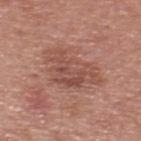Q: Was a biopsy performed?
A: imaged on a skin check; not biopsied
Q: Lesion size?
A: ~7 mm (longest diameter)
Q: What is the imaging modality?
A: ~15 mm tile from a whole-body skin photo
Q: What did automated image analysis measure?
A: a border-irregularity rating of about 6/10, internal color variation of about 4 on a 0–10 scale, and peripheral color asymmetry of about 1.5; a nevus-likeness score of about 0/100 and a detector confidence of about 100 out of 100 that the crop contains a lesion
Q: What lighting was used for the tile?
A: white-light illumination
Q: Lesion location?
A: the upper back
Q: Patient demographics?
A: male, aged around 50